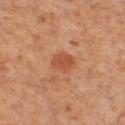Assessment:
The lesion was tiled from a total-body skin photograph and was not biopsied.
Clinical summary:
About 2.5 mm across. A male patient, about 60 years old. Located on the leg. A region of skin cropped from a whole-body photographic capture, roughly 15 mm wide. Imaged with cross-polarized lighting. The total-body-photography lesion software estimated a lesion area of about 5 mm², an outline eccentricity of about 0.5 (0 = round, 1 = elongated), and two-axis asymmetry of about 0.15. It also reported a classifier nevus-likeness of about 55/100.